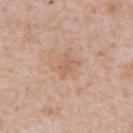Clinical impression: Imaged during a routine full-body skin examination; the lesion was not biopsied and no histopathology is available. Acquisition and patient details: A male subject, aged around 65. On the front of the torso. This image is a 15 mm lesion crop taken from a total-body photograph. This is a white-light tile.